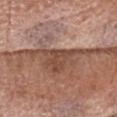No biopsy was performed on this lesion — it was imaged during a full skin examination and was not determined to be concerning.